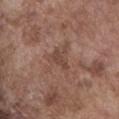{
  "biopsy_status": "not biopsied; imaged during a skin examination",
  "lighting": "white-light",
  "image": {
    "source": "total-body photography crop",
    "field_of_view_mm": 15
  },
  "site": "front of the torso",
  "patient": {
    "sex": "male",
    "age_approx": 75
  }
}